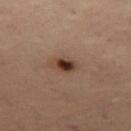Findings:
– follow-up · no biopsy performed (imaged during a skin exam)
– size · ≈3 mm
– subject · female, approximately 55 years of age
– image source · ~15 mm tile from a whole-body skin photo
– body site · the left thigh
– TBP lesion metrics · a lesion color around L≈31 a*≈15 b*≈22 in CIELAB, a lesion–skin lightness drop of about 11, and a lesion-to-skin contrast of about 10.5 (normalized; higher = more distinct); lesion-presence confidence of about 100/100